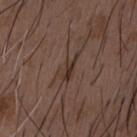Impression:
Recorded during total-body skin imaging; not selected for excision or biopsy.
Acquisition and patient details:
A 15 mm crop from a total-body photograph taken for skin-cancer surveillance. The subject is a male aged approximately 50. Located on the chest.